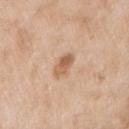Captured during whole-body skin photography for melanoma surveillance; the lesion was not biopsied. Automated tile analysis of the lesion measured a lesion area of about 4 mm², a shape eccentricity near 0.75, and a symmetry-axis asymmetry near 0.25. It also reported a border-irregularity rating of about 2.5/10 and peripheral color asymmetry of about 2. The tile uses white-light illumination. A region of skin cropped from a whole-body photographic capture, roughly 15 mm wide. The subject is a female aged around 40. From the right upper arm.